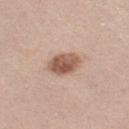Imaged during a routine full-body skin examination; the lesion was not biopsied and no histopathology is available. A female patient, aged 38–42. A lesion tile, about 15 mm wide, cut from a 3D total-body photograph. The lesion is located on the leg. Captured under white-light illumination. Longest diameter approximately 3.5 mm. Automated tile analysis of the lesion measured a border-irregularity index near 1.5/10 and a peripheral color-asymmetry measure near 2.